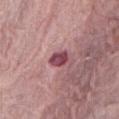- tile lighting: white-light illumination
- automated lesion analysis: a shape eccentricity near 0.65 and a symmetry-axis asymmetry near 0.25; a border-irregularity rating of about 2/10, internal color variation of about 3.5 on a 0–10 scale, and a peripheral color-asymmetry measure near 1
- subject: female, aged 63–67
- size: ≈2.5 mm
- image source: 15 mm crop, total-body photography
- anatomic site: the abdomen